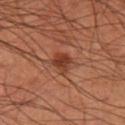follow-up: imaged on a skin check; not biopsied | illumination: cross-polarized illumination | imaging modality: ~15 mm crop, total-body skin-cancer survey | anatomic site: the left forearm | size: about 3 mm | subject: male, aged 58 to 62.